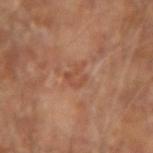workup: total-body-photography surveillance lesion; no biopsy | image source: total-body-photography crop, ~15 mm field of view | TBP lesion metrics: a lesion area of about 4 mm² and a shape eccentricity near 0.7; an automated nevus-likeness rating near 0 out of 100 and lesion-presence confidence of about 100/100 | subject: male, about 65 years old | diameter: ~3 mm (longest diameter) | site: the right forearm | illumination: cross-polarized.